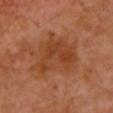biopsy status: catalogued during a skin exam; not biopsied | subject: female, roughly 55 years of age | lighting: cross-polarized illumination | imaging modality: ~15 mm tile from a whole-body skin photo | lesion diameter: ~6.5 mm (longest diameter) | anatomic site: the chest.